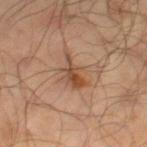Q: Is there a histopathology result?
A: catalogued during a skin exam; not biopsied
Q: Lesion location?
A: the right lower leg
Q: What kind of image is this?
A: ~15 mm tile from a whole-body skin photo
Q: What is the lesion's diameter?
A: ≈4.5 mm
Q: What are the patient's age and sex?
A: male, approximately 65 years of age
Q: What did automated image analysis measure?
A: an area of roughly 7.5 mm², a shape eccentricity near 0.8, and a symmetry-axis asymmetry near 0.4; peripheral color asymmetry of about 1.5; an automated nevus-likeness rating near 70 out of 100 and a lesion-detection confidence of about 100/100
Q: Illumination type?
A: cross-polarized